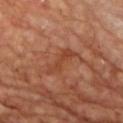The lesion was photographed on a routine skin check and not biopsied; there is no pathology result.
A male subject, aged approximately 55.
Automated image analysis of the tile measured a lesion area of about 6 mm² and a symmetry-axis asymmetry near 0.4. And it measured a lesion color around L≈43 a*≈26 b*≈33 in CIELAB, about 7 CIELAB-L* units darker than the surrounding skin, and a lesion-to-skin contrast of about 5.5 (normalized; higher = more distinct). The analysis additionally found a nevus-likeness score of about 0/100 and a lesion-detection confidence of about 95/100.
Captured under cross-polarized illumination.
Approximately 4.5 mm at its widest.
A lesion tile, about 15 mm wide, cut from a 3D total-body photograph.
The lesion is on the chest.